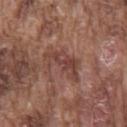Captured during whole-body skin photography for melanoma surveillance; the lesion was not biopsied. The subject is a male aged approximately 75. Located on the front of the torso. Captured under white-light illumination. Approximately 5.5 mm at its widest. Cropped from a total-body skin-imaging series; the visible field is about 15 mm.